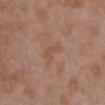Imaged during a routine full-body skin examination; the lesion was not biopsied and no histopathology is available. From the upper back. The subject is a female aged 33–37. A lesion tile, about 15 mm wide, cut from a 3D total-body photograph.